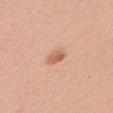biopsy status = no biopsy performed (imaged during a skin exam); lesion size = about 2.5 mm; location = the arm; image = ~15 mm tile from a whole-body skin photo; subject = female, aged approximately 40.